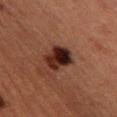notes: catalogued during a skin exam; not biopsied | body site: the left thigh | tile lighting: cross-polarized illumination | size: ≈4 mm | patient: female, aged approximately 40 | automated metrics: a lesion area of about 9 mm² and a symmetry-axis asymmetry near 0.2; border irregularity of about 2.5 on a 0–10 scale, a color-variation rating of about 10/10, and radial color variation of about 3 | image: ~15 mm tile from a whole-body skin photo.